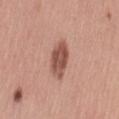notes = catalogued during a skin exam; not biopsied | diameter = ~4.5 mm (longest diameter) | site = the lower back | TBP lesion metrics = a nevus-likeness score of about 30/100 and lesion-presence confidence of about 100/100 | subject = female, about 30 years old | acquisition = ~15 mm crop, total-body skin-cancer survey.